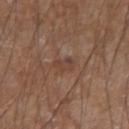Q: Is there a histopathology result?
A: catalogued during a skin exam; not biopsied
Q: Lesion location?
A: the left forearm
Q: What lighting was used for the tile?
A: white-light
Q: What kind of image is this?
A: 15 mm crop, total-body photography
Q: Who is the patient?
A: male, approximately 55 years of age